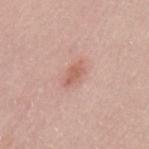The recorded lesion diameter is about 3 mm. The subject is a female aged 28 to 32. Captured under white-light illumination. A lesion tile, about 15 mm wide, cut from a 3D total-body photograph. Located on the upper back. An algorithmic analysis of the crop reported a border-irregularity rating of about 3/10, a within-lesion color-variation index near 1.5/10, and radial color variation of about 0.5.